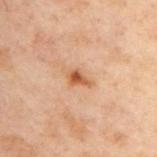lighting: cross-polarized
patient:
  sex: male
  age_approx: 50
image:
  source: total-body photography crop
  field_of_view_mm: 15
site: upper back
automated_metrics:
  area_mm2_approx: 3.0
  eccentricity: 0.85
  shape_asymmetry: 0.4
  vs_skin_darker_L: 11.0
  vs_skin_contrast_norm: 9.0
  color_variation_0_10: 2.0
  peripheral_color_asymmetry: 0.5